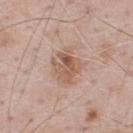The lesion was photographed on a routine skin check and not biopsied; there is no pathology result. The lesion is on the upper back. A male subject, aged 68 to 72. Captured under white-light illumination. Cropped from a total-body skin-imaging series; the visible field is about 15 mm.